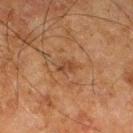Approximately 3 mm at its widest. A 15 mm close-up tile from a total-body photography series done for melanoma screening. On the right thigh. A male patient in their 80s. The tile uses cross-polarized illumination. Automated tile analysis of the lesion measured an area of roughly 4 mm², a shape eccentricity near 0.75, and two-axis asymmetry of about 0.25. And it measured internal color variation of about 2.5 on a 0–10 scale and radial color variation of about 1. The software also gave a lesion-detection confidence of about 100/100.